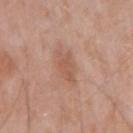Q: Was this lesion biopsied?
A: catalogued during a skin exam; not biopsied
Q: What kind of image is this?
A: ~15 mm tile from a whole-body skin photo
Q: Lesion location?
A: the arm
Q: How was the tile lit?
A: white-light
Q: Patient demographics?
A: male, roughly 65 years of age
Q: What is the lesion's diameter?
A: ~3.5 mm (longest diameter)